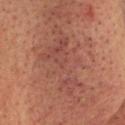<record>
<biopsy_status>not biopsied; imaged during a skin examination</biopsy_status>
<image>
  <source>total-body photography crop</source>
  <field_of_view_mm>15</field_of_view_mm>
</image>
<site>head or neck</site>
<lesion_size>
  <long_diameter_mm_approx>10.0</long_diameter_mm_approx>
</lesion_size>
<patient>
  <sex>male</sex>
  <age_approx>45</age_approx>
</patient>
<lighting>cross-polarized</lighting>
</record>